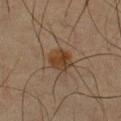Imaged during a routine full-body skin examination; the lesion was not biopsied and no histopathology is available. On the chest. A 15 mm close-up tile from a total-body photography series done for melanoma screening. The patient is a male aged 58 to 62.